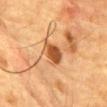Assessment: Recorded during total-body skin imaging; not selected for excision or biopsy. Background: From the chest. About 3.5 mm across. Captured under cross-polarized illumination. A male subject, in their mid- to late 80s or older. Cropped from a total-body skin-imaging series; the visible field is about 15 mm.